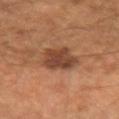| field | value |
|---|---|
| workup | no biopsy performed (imaged during a skin exam) |
| subject | male, approximately 60 years of age |
| tile lighting | cross-polarized illumination |
| imaging modality | 15 mm crop, total-body photography |
| diameter | ≈4.5 mm |
| anatomic site | the left forearm |